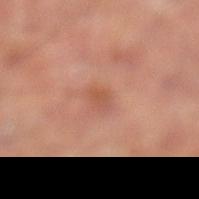Impression:
The lesion was photographed on a routine skin check and not biopsied; there is no pathology result.
Clinical summary:
Imaged with cross-polarized lighting. A male subject, aged 63 to 67. The lesion is on the leg. An algorithmic analysis of the crop reported a lesion color around L≈52 a*≈24 b*≈30 in CIELAB and a lesion–skin lightness drop of about 7. And it measured lesion-presence confidence of about 100/100. A 15 mm close-up extracted from a 3D total-body photography capture. Longest diameter approximately 2.5 mm.